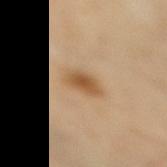site: the right lower leg
patient: female, in their 50s
image: total-body-photography crop, ~15 mm field of view
tile lighting: cross-polarized illumination
image-analysis metrics: a lesion area of about 6 mm², a shape eccentricity near 0.8, and a symmetry-axis asymmetry near 0.2; a lesion–skin lightness drop of about 11 and a normalized border contrast of about 8.5; a border-irregularity rating of about 2/10, internal color variation of about 3 on a 0–10 scale, and a peripheral color-asymmetry measure near 0.5